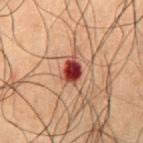Assessment:
This lesion was catalogued during total-body skin photography and was not selected for biopsy.
Image and clinical context:
Automated image analysis of the tile measured an area of roughly 5.5 mm² and a symmetry-axis asymmetry near 0.25. And it measured an average lesion color of about L≈32 a*≈26 b*≈23 (CIELAB) and a normalized border contrast of about 14. And it measured a border-irregularity index near 2.5/10, internal color variation of about 6.5 on a 0–10 scale, and a peripheral color-asymmetry measure near 2. Approximately 3 mm at its widest. The lesion is located on the abdomen. The tile uses cross-polarized illumination. Cropped from a whole-body photographic skin survey; the tile spans about 15 mm. A male patient roughly 50 years of age.